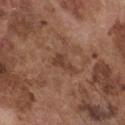Assessment:
The lesion was tiled from a total-body skin photograph and was not biopsied.
Acquisition and patient details:
From the front of the torso. A male subject in their mid- to late 70s. A 15 mm crop from a total-body photograph taken for skin-cancer surveillance. The lesion's longest dimension is about 3 mm. Automated tile analysis of the lesion measured a footprint of about 3.5 mm² and two-axis asymmetry of about 0.5. The analysis additionally found a border-irregularity rating of about 5/10, internal color variation of about 1 on a 0–10 scale, and radial color variation of about 0.5.